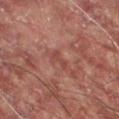{
  "biopsy_status": "not biopsied; imaged during a skin examination",
  "lighting": "cross-polarized",
  "image": {
    "source": "total-body photography crop",
    "field_of_view_mm": 15
  },
  "patient": {
    "sex": "male",
    "age_approx": 55
  },
  "lesion_size": {
    "long_diameter_mm_approx": 2.5
  },
  "automated_metrics": {
    "border_irregularity_0_10": 5.0,
    "color_variation_0_10": 0.0
  },
  "site": "chest"
}